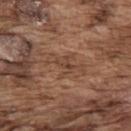biopsy status — no biopsy performed (imaged during a skin exam); patient — male, roughly 75 years of age; anatomic site — the back; diameter — ~2.5 mm (longest diameter); acquisition — ~15 mm tile from a whole-body skin photo.